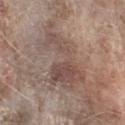The lesion was photographed on a routine skin check and not biopsied; there is no pathology result. On the right forearm. This is a white-light tile. A female subject, roughly 75 years of age. A 15 mm close-up tile from a total-body photography series done for melanoma screening. Measured at roughly 8.5 mm in maximum diameter.